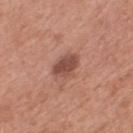Acquisition and patient details: A male patient roughly 55 years of age. A 15 mm close-up tile from a total-body photography series done for melanoma screening. From the mid back.